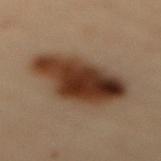Q: Is there a histopathology result?
A: catalogued during a skin exam; not biopsied